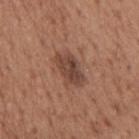Imaged during a routine full-body skin examination; the lesion was not biopsied and no histopathology is available.